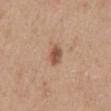The lesion was tiled from a total-body skin photograph and was not biopsied.
A male patient aged around 65.
An algorithmic analysis of the crop reported an area of roughly 4 mm² and two-axis asymmetry of about 0.15. The analysis additionally found a border-irregularity rating of about 1.5/10, a within-lesion color-variation index near 4/10, and a peripheral color-asymmetry measure near 1.5.
The lesion is on the mid back.
Cropped from a total-body skin-imaging series; the visible field is about 15 mm.
The recorded lesion diameter is about 2.5 mm.
This is a white-light tile.